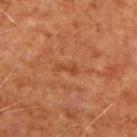{
  "patient": {
    "sex": "male",
    "age_approx": 70
  },
  "site": "arm",
  "lesion_size": {
    "long_diameter_mm_approx": 2.5
  },
  "image": {
    "source": "total-body photography crop",
    "field_of_view_mm": 15
  }
}